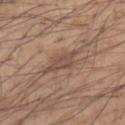workup — catalogued during a skin exam; not biopsied
imaging modality — ~15 mm tile from a whole-body skin photo
tile lighting — white-light illumination
site — the left forearm
lesion size — about 5.5 mm
subject — male, aged 53 to 57